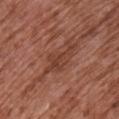Q: Was this lesion biopsied?
A: catalogued during a skin exam; not biopsied
Q: How large is the lesion?
A: ≈5 mm
Q: How was this image acquired?
A: total-body-photography crop, ~15 mm field of view
Q: What is the anatomic site?
A: the front of the torso
Q: How was the tile lit?
A: white-light
Q: What are the patient's age and sex?
A: male, about 75 years old